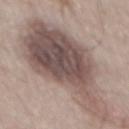Notes:
* follow-up · catalogued during a skin exam; not biopsied
* imaging modality · total-body-photography crop, ~15 mm field of view
* image-analysis metrics · a mean CIELAB color near L≈51 a*≈14 b*≈19 and a lesion–skin lightness drop of about 14; internal color variation of about 7 on a 0–10 scale and a peripheral color-asymmetry measure near 2; a nevus-likeness score of about 90/100
* lighting · white-light illumination
* patient · male, aged approximately 55
* size · about 14.5 mm
* site · the back